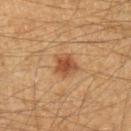This lesion was catalogued during total-body skin photography and was not selected for biopsy. The patient is a male aged 38–42. A roughly 15 mm field-of-view crop from a total-body skin photograph. Located on the left forearm.